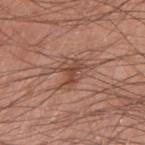biopsy status = imaged on a skin check; not biopsied
imaging modality = total-body-photography crop, ~15 mm field of view
patient = male, aged around 45
site = the left upper arm